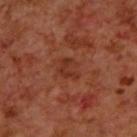No biopsy was performed on this lesion — it was imaged during a full skin examination and was not determined to be concerning.
The patient is a male aged around 70.
Cropped from a total-body skin-imaging series; the visible field is about 15 mm.
This is a cross-polarized tile.
Located on the upper back.
About 3 mm across.